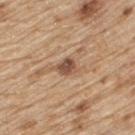Captured during whole-body skin photography for melanoma surveillance; the lesion was not biopsied. On the back. A male patient, roughly 70 years of age. A region of skin cropped from a whole-body photographic capture, roughly 15 mm wide.